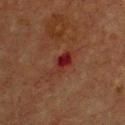Impression:
The lesion was tiled from a total-body skin photograph and was not biopsied.
Image and clinical context:
The patient is a male aged 73 to 77. From the front of the torso. Cropped from a whole-body photographic skin survey; the tile spans about 15 mm.